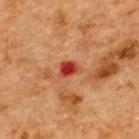– notes: total-body-photography surveillance lesion; no biopsy
– lesion diameter: ~2.5 mm (longest diameter)
– lighting: cross-polarized
– patient: male, aged 63 to 67
– imaging modality: ~15 mm crop, total-body skin-cancer survey
– TBP lesion metrics: a footprint of about 4 mm² and two-axis asymmetry of about 0.2; a mean CIELAB color near L≈37 a*≈33 b*≈32, a lesion–skin lightness drop of about 12, and a normalized lesion–skin contrast near 10
– anatomic site: the upper back